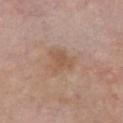No biopsy was performed on this lesion — it was imaged during a full skin examination and was not determined to be concerning.
A 15 mm close-up tile from a total-body photography series done for melanoma screening.
Imaged with white-light lighting.
A male patient aged 63–67.
The recorded lesion diameter is about 3 mm.
The total-body-photography lesion software estimated an area of roughly 6 mm² and an eccentricity of roughly 0.6.
Located on the chest.